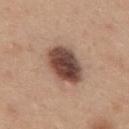The lesion was tiled from a total-body skin photograph and was not biopsied. Captured under white-light illumination. The patient is a male aged 33 to 37. The lesion is on the mid back. Automated tile analysis of the lesion measured an area of roughly 14 mm². A 15 mm close-up tile from a total-body photography series done for melanoma screening.